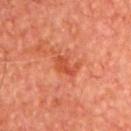Assessment: The lesion was photographed on a routine skin check and not biopsied; there is no pathology result. Acquisition and patient details: A male patient, about 65 years old. Captured under cross-polarized illumination. Located on the chest. A 15 mm close-up extracted from a 3D total-body photography capture.